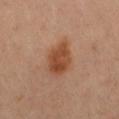Part of a total-body skin-imaging series; this lesion was reviewed on a skin check and was not flagged for biopsy. The lesion-visualizer software estimated a footprint of about 9.5 mm² and an outline eccentricity of about 0.7 (0 = round, 1 = elongated). The software also gave an average lesion color of about L≈45 a*≈24 b*≈33 (CIELAB), a lesion–skin lightness drop of about 11, and a normalized border contrast of about 9. The analysis additionally found a border-irregularity rating of about 2/10, internal color variation of about 3 on a 0–10 scale, and radial color variation of about 1. And it measured an automated nevus-likeness rating near 100 out of 100. This is a cross-polarized tile. A 15 mm crop from a total-body photograph taken for skin-cancer surveillance. A female patient, roughly 50 years of age. Located on the right thigh. Longest diameter approximately 4.5 mm.